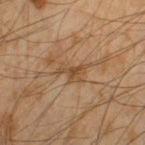Case summary:
• size · about 3 mm
• subject · male, aged 43 to 47
• lighting · cross-polarized
• image · 15 mm crop, total-body photography
• anatomic site · the left thigh